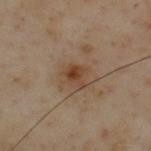The lesion was tiled from a total-body skin photograph and was not biopsied.
The tile uses cross-polarized illumination.
A male subject, aged around 55.
About 3 mm across.
On the upper back.
A lesion tile, about 15 mm wide, cut from a 3D total-body photograph.
Automated image analysis of the tile measured an average lesion color of about L≈43 a*≈18 b*≈30 (CIELAB) and roughly 8 lightness units darker than nearby skin. The software also gave a border-irregularity index near 3.5/10, a color-variation rating of about 6/10, and peripheral color asymmetry of about 2. And it measured a nevus-likeness score of about 30/100.